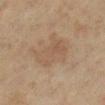Findings:
* tile lighting · cross-polarized
* acquisition · ~15 mm crop, total-body skin-cancer survey
* subject · female, roughly 40 years of age
* size · about 5.5 mm
* site · the left lower leg